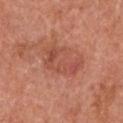Impression:
Part of a total-body skin-imaging series; this lesion was reviewed on a skin check and was not flagged for biopsy.
Clinical summary:
The subject is a male about 65 years old. The lesion is on the left upper arm. A 15 mm crop from a total-body photograph taken for skin-cancer surveillance. This is a white-light tile. The lesion's longest dimension is about 5 mm.